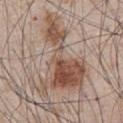Clinical impression: Imaged during a routine full-body skin examination; the lesion was not biopsied and no histopathology is available. Context: Approximately 10.5 mm at its widest. Located on the front of the torso. The tile uses white-light illumination. This image is a 15 mm lesion crop taken from a total-body photograph. A male patient in their mid- to late 60s.